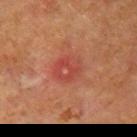Q: Patient demographics?
A: male, aged 63–67
Q: Where on the body is the lesion?
A: the upper back
Q: How was this image acquired?
A: ~15 mm tile from a whole-body skin photo